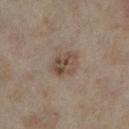No biopsy was performed on this lesion — it was imaged during a full skin examination and was not determined to be concerning. A close-up tile cropped from a whole-body skin photograph, about 15 mm across. A female patient in their mid-30s. From the leg.